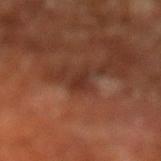<case>
  <site>leg</site>
  <image>
    <source>total-body photography crop</source>
    <field_of_view_mm>15</field_of_view_mm>
  </image>
  <lighting>cross-polarized</lighting>
  <patient>
    <sex>male</sex>
    <age_approx>65</age_approx>
  </patient>
</case>